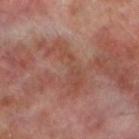Q: Is there a histopathology result?
A: imaged on a skin check; not biopsied
Q: Who is the patient?
A: male, aged 68–72
Q: How was the tile lit?
A: cross-polarized
Q: What did automated image analysis measure?
A: a lesion area of about 8.5 mm², an outline eccentricity of about 0.95 (0 = round, 1 = elongated), and a shape-asymmetry score of about 0.65 (0 = symmetric); a border-irregularity index near 10/10 and a within-lesion color-variation index near 2/10
Q: How was this image acquired?
A: 15 mm crop, total-body photography
Q: Lesion location?
A: the left lower leg
Q: Lesion size?
A: ~6 mm (longest diameter)